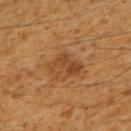body site = the upper back
subject = male, aged 58 to 62
acquisition = ~15 mm tile from a whole-body skin photo